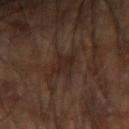Context:
Captured under cross-polarized illumination. A 15 mm close-up tile from a total-body photography series done for melanoma screening. On the right arm. A male subject roughly 60 years of age.